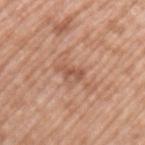| field | value |
|---|---|
| follow-up | total-body-photography surveillance lesion; no biopsy |